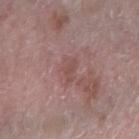No biopsy was performed on this lesion — it was imaged during a full skin examination and was not determined to be concerning. Longest diameter approximately 2.5 mm. Imaged with white-light lighting. This image is a 15 mm lesion crop taken from a total-body photograph. The total-body-photography lesion software estimated a within-lesion color-variation index near 1/10. The lesion is located on the right forearm. The patient is a female in their mid-60s.